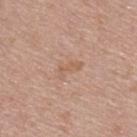Captured during whole-body skin photography for melanoma surveillance; the lesion was not biopsied.
A roughly 15 mm field-of-view crop from a total-body skin photograph.
The subject is a male aged around 55.
Measured at roughly 3 mm in maximum diameter.
The total-body-photography lesion software estimated radial color variation of about 0.5.
Captured under white-light illumination.